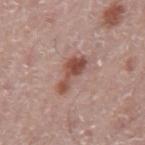Imaged during a routine full-body skin examination; the lesion was not biopsied and no histopathology is available. The lesion-visualizer software estimated a footprint of about 7.5 mm², an outline eccentricity of about 0.9 (0 = round, 1 = elongated), and a symmetry-axis asymmetry near 0.5. The lesion is on the mid back. This is a white-light tile. Cropped from a total-body skin-imaging series; the visible field is about 15 mm. A male patient, aged approximately 75.